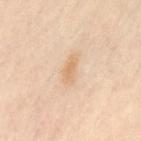This lesion was catalogued during total-body skin photography and was not selected for biopsy. Approximately 3 mm at its widest. The lesion is located on the lower back. A female subject, aged 38–42. Automated tile analysis of the lesion measured a border-irregularity index near 3/10 and peripheral color asymmetry of about 0. It also reported a nevus-likeness score of about 5/100 and a detector confidence of about 100 out of 100 that the crop contains a lesion. A close-up tile cropped from a whole-body skin photograph, about 15 mm across.